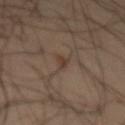This is a cross-polarized tile. Automated image analysis of the tile measured a footprint of about 2.5 mm². The software also gave a lesion color around L≈37 a*≈15 b*≈23 in CIELAB, roughly 7 lightness units darker than nearby skin, and a normalized lesion–skin contrast near 6.5. The software also gave a border-irregularity rating of about 4/10, internal color variation of about 0 on a 0–10 scale, and peripheral color asymmetry of about 0. It also reported a classifier nevus-likeness of about 80/100 and a detector confidence of about 100 out of 100 that the crop contains a lesion. A male subject, approximately 60 years of age. A close-up tile cropped from a whole-body skin photograph, about 15 mm across. From the front of the torso.